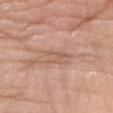This lesion was catalogued during total-body skin photography and was not selected for biopsy. The total-body-photography lesion software estimated a footprint of about 4 mm² and an outline eccentricity of about 0.75 (0 = round, 1 = elongated). The analysis additionally found a border-irregularity rating of about 3/10 and a peripheral color-asymmetry measure near 1. And it measured an automated nevus-likeness rating near 0 out of 100 and lesion-presence confidence of about 65/100. The tile uses white-light illumination. A male subject aged approximately 60. The lesion is on the left forearm. A close-up tile cropped from a whole-body skin photograph, about 15 mm across.